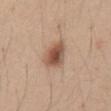The lesion was tiled from a total-body skin photograph and was not biopsied. A 15 mm close-up extracted from a 3D total-body photography capture. Located on the abdomen. Automated tile analysis of the lesion measured a normalized lesion–skin contrast near 9. And it measured border irregularity of about 2 on a 0–10 scale, internal color variation of about 5.5 on a 0–10 scale, and peripheral color asymmetry of about 1.5. Captured under white-light illumination. A male subject, roughly 35 years of age. Measured at roughly 4 mm in maximum diameter.